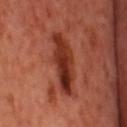Assessment:
This lesion was catalogued during total-body skin photography and was not selected for biopsy.
Image and clinical context:
On the head or neck. A male patient aged approximately 55. Imaged with cross-polarized lighting. The total-body-photography lesion software estimated a classifier nevus-likeness of about 85/100 and a lesion-detection confidence of about 100/100. This image is a 15 mm lesion crop taken from a total-body photograph.